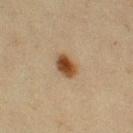The lesion was tiled from a total-body skin photograph and was not biopsied. The tile uses cross-polarized illumination. Located on the left thigh. A female patient, in their mid- to late 50s. Approximately 3 mm at its widest. A region of skin cropped from a whole-body photographic capture, roughly 15 mm wide.